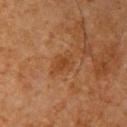Captured during whole-body skin photography for melanoma surveillance; the lesion was not biopsied.
Located on the right upper arm.
The total-body-photography lesion software estimated an area of roughly 5.5 mm² and an eccentricity of roughly 0.7. And it measured a lesion color around L≈34 a*≈19 b*≈31 in CIELAB, a lesion–skin lightness drop of about 6, and a normalized border contrast of about 6. It also reported an automated nevus-likeness rating near 0 out of 100 and lesion-presence confidence of about 100/100.
About 3 mm across.
The patient is a male roughly 65 years of age.
This image is a 15 mm lesion crop taken from a total-body photograph.
Captured under cross-polarized illumination.